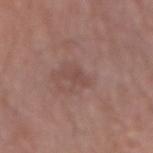On the right forearm.
Cropped from a total-body skin-imaging series; the visible field is about 15 mm.
A male subject, aged approximately 75.
Automated tile analysis of the lesion measured an area of roughly 3 mm², an outline eccentricity of about 0.9 (0 = round, 1 = elongated), and a symmetry-axis asymmetry near 0.4. And it measured an average lesion color of about L≈47 a*≈19 b*≈23 (CIELAB) and a normalized border contrast of about 5. It also reported a within-lesion color-variation index near 1/10 and peripheral color asymmetry of about 0.
Measured at roughly 3 mm in maximum diameter.
Imaged with white-light lighting.